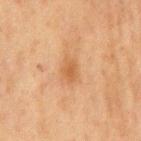Notes:
• follow-up · total-body-photography surveillance lesion; no biopsy
• tile lighting · cross-polarized
• automated lesion analysis · a lesion color around L≈47 a*≈19 b*≈34 in CIELAB and about 7 CIELAB-L* units darker than the surrounding skin; internal color variation of about 2 on a 0–10 scale and radial color variation of about 0.5
• body site · the back
• imaging modality · total-body-photography crop, ~15 mm field of view
• lesion size · ≈3 mm
• subject · male, aged 73–77